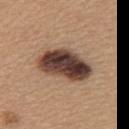Recorded during total-body skin imaging; not selected for excision or biopsy.
The lesion is located on the upper back.
The lesion-visualizer software estimated a lesion area of about 21 mm², an eccentricity of roughly 0.85, and a shape-asymmetry score of about 0.2 (0 = symmetric). The software also gave a mean CIELAB color near L≈41 a*≈17 b*≈24, a lesion–skin lightness drop of about 21, and a normalized lesion–skin contrast near 15.5. The analysis additionally found a classifier nevus-likeness of about 95/100 and a detector confidence of about 100 out of 100 that the crop contains a lesion.
Approximately 7 mm at its widest.
A 15 mm close-up tile from a total-body photography series done for melanoma screening.
The tile uses white-light illumination.
The patient is a female approximately 60 years of age.